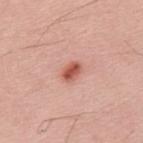<record>
  <biopsy_status>not biopsied; imaged during a skin examination</biopsy_status>
  <site>back</site>
  <image>
    <source>total-body photography crop</source>
    <field_of_view_mm>15</field_of_view_mm>
  </image>
  <patient>
    <sex>male</sex>
    <age_approx>30</age_approx>
  </patient>
</record>